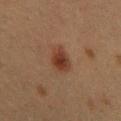This lesion was catalogued during total-body skin photography and was not selected for biopsy. The tile uses cross-polarized illumination. From the upper back. Cropped from a whole-body photographic skin survey; the tile spans about 15 mm. A female patient, aged approximately 40.